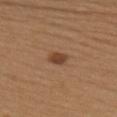Notes:
– notes — no biopsy performed (imaged during a skin exam)
– lighting — white-light illumination
– automated lesion analysis — a mean CIELAB color near L≈43 a*≈21 b*≈31 and a lesion-to-skin contrast of about 8.5 (normalized; higher = more distinct); a classifier nevus-likeness of about 95/100
– location — the upper back
– image source — total-body-photography crop, ~15 mm field of view
– subject — female, roughly 40 years of age